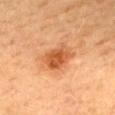biopsy status = catalogued during a skin exam; not biopsied | image-analysis metrics = a border-irregularity rating of about 2.5/10, a color-variation rating of about 4/10, and peripheral color asymmetry of about 1 | patient = female, aged around 60 | anatomic site = the back | image = 15 mm crop, total-body photography | size = ~4.5 mm (longest diameter).